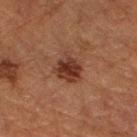This lesion was catalogued during total-body skin photography and was not selected for biopsy. The subject is a male aged 73–77. Located on the right forearm. A 15 mm close-up tile from a total-body photography series done for melanoma screening.